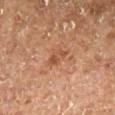biopsy status: imaged on a skin check; not biopsied.